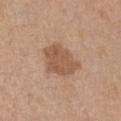anatomic site = the abdomen | acquisition = 15 mm crop, total-body photography | lesion diameter = about 4.5 mm | tile lighting = white-light | subject = female, in their mid- to late 60s.